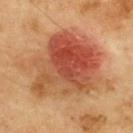Recorded during total-body skin imaging; not selected for excision or biopsy.
A male subject, aged approximately 70.
Automated image analysis of the tile measured a footprint of about 55 mm², a shape eccentricity near 0.6, and two-axis asymmetry of about 0.3. It also reported a mean CIELAB color near L≈50 a*≈26 b*≈35, roughly 13 lightness units darker than nearby skin, and a normalized lesion–skin contrast near 9. And it measured an automated nevus-likeness rating near 100 out of 100.
On the upper back.
A roughly 15 mm field-of-view crop from a total-body skin photograph.
Approximately 11 mm at its widest.
Captured under cross-polarized illumination.